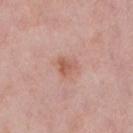Clinical impression: This lesion was catalogued during total-body skin photography and was not selected for biopsy. Clinical summary: A female patient, in their 40s. A lesion tile, about 15 mm wide, cut from a 3D total-body photograph. On the right lower leg. The lesion-visualizer software estimated a footprint of about 4 mm², an eccentricity of roughly 0.5, and a shape-asymmetry score of about 0.4 (0 = symmetric). The software also gave a border-irregularity index near 3.5/10, internal color variation of about 2.5 on a 0–10 scale, and peripheral color asymmetry of about 1. The software also gave a nevus-likeness score of about 60/100 and lesion-presence confidence of about 100/100.